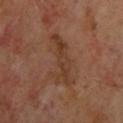Q: Is there a histopathology result?
A: imaged on a skin check; not biopsied